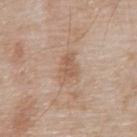A male subject, aged 78–82.
The lesion's longest dimension is about 4 mm.
The lesion is on the abdomen.
A roughly 15 mm field-of-view crop from a total-body skin photograph.